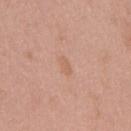Clinical summary:
A female subject, roughly 40 years of age. The total-body-photography lesion software estimated a lesion color around L≈61 a*≈21 b*≈31 in CIELAB, about 6 CIELAB-L* units darker than the surrounding skin, and a normalized lesion–skin contrast near 4.5. And it measured a nevus-likeness score of about 0/100 and a lesion-detection confidence of about 100/100. This is a white-light tile. A 15 mm close-up extracted from a 3D total-body photography capture. The lesion is on the back.